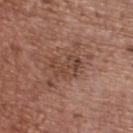| feature | finding |
|---|---|
| lesion diameter | ≈4 mm |
| patient | female, roughly 65 years of age |
| body site | the upper back |
| tile lighting | white-light illumination |
| imaging modality | 15 mm crop, total-body photography |